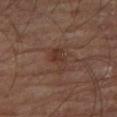Findings:
• follow-up — imaged on a skin check; not biopsied
• illumination — cross-polarized illumination
• size — ≈3.5 mm
• location — the leg
• image source — total-body-photography crop, ~15 mm field of view
• patient — male, approximately 65 years of age